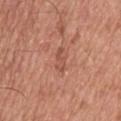| field | value |
|---|---|
| workup | catalogued during a skin exam; not biopsied |
| imaging modality | ~15 mm crop, total-body skin-cancer survey |
| location | the upper back |
| automated lesion analysis | a footprint of about 3.5 mm² and an eccentricity of roughly 0.9; a lesion color around L≈52 a*≈26 b*≈30 in CIELAB and about 7 CIELAB-L* units darker than the surrounding skin; a border-irregularity index near 3.5/10, a color-variation rating of about 1/10, and a peripheral color-asymmetry measure near 0 |
| size | about 3 mm |
| patient | male, aged approximately 55 |
| illumination | white-light illumination |